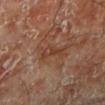biopsy status: catalogued during a skin exam; not biopsied | illumination: cross-polarized illumination | location: the left lower leg | diameter: ≈3.5 mm | subject: male, aged 68 to 72 | acquisition: 15 mm crop, total-body photography.